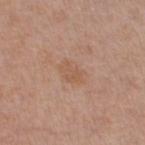{
  "patient": {
    "sex": "female",
    "age_approx": 55
  },
  "lighting": "white-light",
  "site": "left thigh",
  "lesion_size": {
    "long_diameter_mm_approx": 3.0
  },
  "automated_metrics": {
    "area_mm2_approx": 6.5,
    "eccentricity": 0.6,
    "cielab_L": 56,
    "cielab_a": 19,
    "cielab_b": 31,
    "vs_skin_darker_L": 5.0,
    "nevus_likeness_0_100": 0,
    "lesion_detection_confidence_0_100": 100
  },
  "image": {
    "source": "total-body photography crop",
    "field_of_view_mm": 15
  }
}